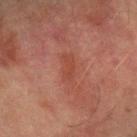Q: Is there a histopathology result?
A: imaged on a skin check; not biopsied
Q: Lesion location?
A: the left forearm
Q: What is the imaging modality?
A: 15 mm crop, total-body photography
Q: Who is the patient?
A: male, aged 73 to 77
Q: What is the lesion's diameter?
A: about 3.5 mm